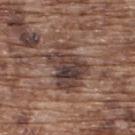Clinical impression:
This lesion was catalogued during total-body skin photography and was not selected for biopsy.
Acquisition and patient details:
A male patient, aged 73 to 77. An algorithmic analysis of the crop reported a mean CIELAB color near L≈40 a*≈16 b*≈21, a lesion–skin lightness drop of about 12, and a lesion-to-skin contrast of about 10 (normalized; higher = more distinct). And it measured border irregularity of about 5 on a 0–10 scale, internal color variation of about 7.5 on a 0–10 scale, and a peripheral color-asymmetry measure near 2.5. A region of skin cropped from a whole-body photographic capture, roughly 15 mm wide. The lesion is on the upper back. Captured under white-light illumination. The recorded lesion diameter is about 6 mm.